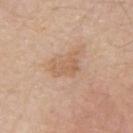Impression:
Captured during whole-body skin photography for melanoma surveillance; the lesion was not biopsied.
Image and clinical context:
From the left arm. A male subject in their 80s. Cropped from a whole-body photographic skin survey; the tile spans about 15 mm.